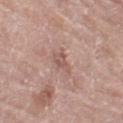Notes:
• automated metrics · a lesion area of about 3.5 mm², an outline eccentricity of about 0.85 (0 = round, 1 = elongated), and a shape-asymmetry score of about 0.35 (0 = symmetric); a lesion–skin lightness drop of about 8 and a lesion-to-skin contrast of about 5.5 (normalized; higher = more distinct)
• site · the left thigh
• imaging modality · ~15 mm crop, total-body skin-cancer survey
• tile lighting · white-light illumination
• subject · female, aged 58–62
• size · ≈3 mm